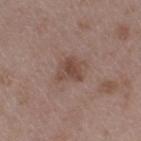{
  "biopsy_status": "not biopsied; imaged during a skin examination",
  "lesion_size": {
    "long_diameter_mm_approx": 3.0
  },
  "site": "left thigh",
  "patient": {
    "sex": "male",
    "age_approx": 50
  },
  "lighting": "white-light",
  "image": {
    "source": "total-body photography crop",
    "field_of_view_mm": 15
  }
}